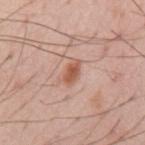workup: imaged on a skin check; not biopsied
image source: 15 mm crop, total-body photography
body site: the mid back
subject: male, roughly 55 years of age